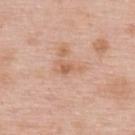This lesion was catalogued during total-body skin photography and was not selected for biopsy.
The recorded lesion diameter is about 3 mm.
Captured under white-light illumination.
A female subject, roughly 50 years of age.
A 15 mm close-up extracted from a 3D total-body photography capture.
Located on the back.